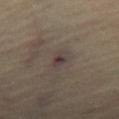Approximately 3 mm at its widest.
The lesion-visualizer software estimated an area of roughly 3.5 mm², an outline eccentricity of about 0.85 (0 = round, 1 = elongated), and a symmetry-axis asymmetry near 0.25.
The lesion is on the right thigh.
The tile uses cross-polarized illumination.
A roughly 15 mm field-of-view crop from a total-body skin photograph.
A female patient, approximately 65 years of age.